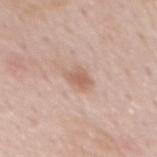Q: Was a biopsy performed?
A: total-body-photography surveillance lesion; no biopsy
Q: Lesion location?
A: the mid back
Q: How was this image acquired?
A: total-body-photography crop, ~15 mm field of view
Q: What are the patient's age and sex?
A: male, about 55 years old
Q: What did automated image analysis measure?
A: a shape eccentricity near 0.65 and two-axis asymmetry of about 0.3; an average lesion color of about L≈61 a*≈20 b*≈28 (CIELAB), roughly 9 lightness units darker than nearby skin, and a lesion-to-skin contrast of about 6.5 (normalized; higher = more distinct)